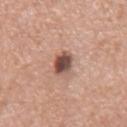Assessment:
Part of a total-body skin-imaging series; this lesion was reviewed on a skin check and was not flagged for biopsy.
Image and clinical context:
Located on the mid back. Automated tile analysis of the lesion measured a footprint of about 5.5 mm², a shape eccentricity near 0.65, and a shape-asymmetry score of about 0.15 (0 = symmetric). The software also gave a border-irregularity rating of about 1.5/10, internal color variation of about 6 on a 0–10 scale, and peripheral color asymmetry of about 2. The patient is a male aged 73–77. The lesion's longest dimension is about 3 mm. A 15 mm crop from a total-body photograph taken for skin-cancer surveillance.